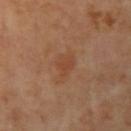Impression:
Part of a total-body skin-imaging series; this lesion was reviewed on a skin check and was not flagged for biopsy.
Background:
A female patient, about 60 years old. Imaged with cross-polarized lighting. Automated tile analysis of the lesion measured about 6 CIELAB-L* units darker than the surrounding skin and a lesion-to-skin contrast of about 5 (normalized; higher = more distinct). The analysis additionally found a border-irregularity rating of about 3.5/10 and a peripheral color-asymmetry measure near 0.5. And it measured lesion-presence confidence of about 100/100. From the arm. This image is a 15 mm lesion crop taken from a total-body photograph. Longest diameter approximately 3.5 mm.